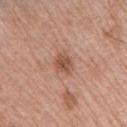This lesion was catalogued during total-body skin photography and was not selected for biopsy.